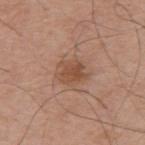The lesion was tiled from a total-body skin photograph and was not biopsied.
A male subject, roughly 75 years of age.
The lesion is located on the right upper arm.
The tile uses white-light illumination.
A 15 mm close-up tile from a total-body photography series done for melanoma screening.
Automated tile analysis of the lesion measured a lesion area of about 6.5 mm² and a shape-asymmetry score of about 0.25 (0 = symmetric).